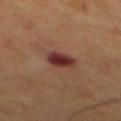– diameter — ≈3 mm
– imaging modality — ~15 mm crop, total-body skin-cancer survey
– anatomic site — the mid back
– patient — male, roughly 65 years of age
– lighting — cross-polarized